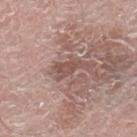follow-up: total-body-photography surveillance lesion; no biopsy | patient: male, aged around 70 | imaging modality: total-body-photography crop, ~15 mm field of view | size: about 4 mm | location: the right lower leg | lighting: white-light.